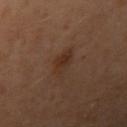| key | value |
|---|---|
| workup | imaged on a skin check; not biopsied |
| image-analysis metrics | a footprint of about 6 mm² and an eccentricity of roughly 0.75; border irregularity of about 3.5 on a 0–10 scale and internal color variation of about 3.5 on a 0–10 scale; a detector confidence of about 100 out of 100 that the crop contains a lesion |
| subject | male, approximately 40 years of age |
| image source | total-body-photography crop, ~15 mm field of view |
| anatomic site | the right upper arm |
| lighting | cross-polarized illumination |